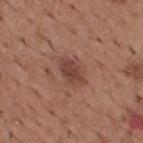The lesion's longest dimension is about 3.5 mm. On the upper back. The lesion-visualizer software estimated a lesion color around L≈43 a*≈22 b*≈24 in CIELAB. The software also gave a classifier nevus-likeness of about 60/100. A male patient aged approximately 65. A region of skin cropped from a whole-body photographic capture, roughly 15 mm wide.